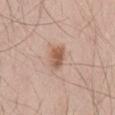<case>
<biopsy_status>not biopsied; imaged during a skin examination</biopsy_status>
<image>
  <source>total-body photography crop</source>
  <field_of_view_mm>15</field_of_view_mm>
</image>
<patient>
  <sex>male</sex>
  <age_approx>45</age_approx>
</patient>
<site>lower back</site>
<lighting>white-light</lighting>
</case>